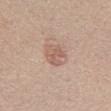Notes:
• lesion size: ≈3.5 mm
• body site: the chest
• image: total-body-photography crop, ~15 mm field of view
• lighting: white-light illumination
• patient: female, in their mid-60s